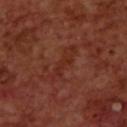The lesion was tiled from a total-body skin photograph and was not biopsied. From the upper back. A lesion tile, about 15 mm wide, cut from a 3D total-body photograph. The tile uses cross-polarized illumination. The lesion's longest dimension is about 5 mm. A male subject approximately 70 years of age.